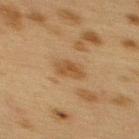Recorded during total-body skin imaging; not selected for excision or biopsy. The total-body-photography lesion software estimated a footprint of about 5 mm² and two-axis asymmetry of about 0.2. The analysis additionally found an average lesion color of about L≈42 a*≈16 b*≈32 (CIELAB), about 7 CIELAB-L* units darker than the surrounding skin, and a normalized lesion–skin contrast near 7. It also reported border irregularity of about 2 on a 0–10 scale, a within-lesion color-variation index near 2/10, and radial color variation of about 0.5. It also reported an automated nevus-likeness rating near 5 out of 100. The subject is a female aged 38 to 42. A 15 mm close-up tile from a total-body photography series done for melanoma screening. Located on the upper back.